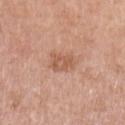biopsy_status: not biopsied; imaged during a skin examination
automated_metrics:
  area_mm2_approx: 5.0
  eccentricity: 0.5
  shape_asymmetry: 0.2
  cielab_L: 57
  cielab_a: 23
  cielab_b: 32
  vs_skin_darker_L: 8.0
  vs_skin_contrast_norm: 6.0
patient:
  sex: female
  age_approx: 70
site: left upper arm
lighting: white-light
image:
  source: total-body photography crop
  field_of_view_mm: 15